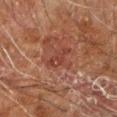Case summary:
• tile lighting — cross-polarized
• lesion size — ~3 mm (longest diameter)
• image source — total-body-photography crop, ~15 mm field of view
• body site — the left arm
• patient — male, roughly 60 years of age